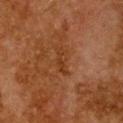Part of a total-body skin-imaging series; this lesion was reviewed on a skin check and was not flagged for biopsy.
A roughly 15 mm field-of-view crop from a total-body skin photograph.
Imaged with cross-polarized lighting.
A female subject aged 48–52.
On the chest.